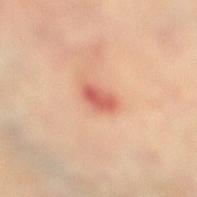{"biopsy_status": "not biopsied; imaged during a skin examination", "site": "leg", "automated_metrics": {"area_mm2_approx": 4.5, "cielab_L": 62, "cielab_a": 32, "cielab_b": 33, "vs_skin_darker_L": 13.0, "vs_skin_contrast_norm": 8.0, "border_irregularity_0_10": 2.0, "color_variation_0_10": 3.0, "peripheral_color_asymmetry": 1.0, "nevus_likeness_0_100": 0, "lesion_detection_confidence_0_100": 100}, "image": {"source": "total-body photography crop", "field_of_view_mm": 15}, "lesion_size": {"long_diameter_mm_approx": 3.0}, "patient": {"sex": "female", "age_approx": 65}}